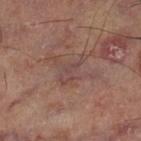workup: total-body-photography surveillance lesion; no biopsy
body site: the left thigh
image source: ~15 mm crop, total-body skin-cancer survey
lesion size: ≈4 mm